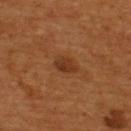This lesion was catalogued during total-body skin photography and was not selected for biopsy.
The lesion is located on the back.
A lesion tile, about 15 mm wide, cut from a 3D total-body photograph.
A male patient, aged approximately 55.